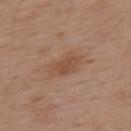Q: Was this lesion biopsied?
A: total-body-photography surveillance lesion; no biopsy
Q: What did automated image analysis measure?
A: an eccentricity of roughly 0.85 and a shape-asymmetry score of about 0.2 (0 = symmetric); a border-irregularity index near 2.5/10, a color-variation rating of about 2.5/10, and peripheral color asymmetry of about 0.5; an automated nevus-likeness rating near 5 out of 100 and a lesion-detection confidence of about 100/100
Q: What is the anatomic site?
A: the back
Q: Lesion size?
A: about 4.5 mm
Q: How was this image acquired?
A: total-body-photography crop, ~15 mm field of view
Q: How was the tile lit?
A: white-light
Q: Patient demographics?
A: male, roughly 55 years of age